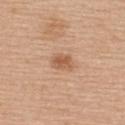Impression:
This lesion was catalogued during total-body skin photography and was not selected for biopsy.
Background:
The total-body-photography lesion software estimated an average lesion color of about L≈58 a*≈21 b*≈34 (CIELAB), roughly 10 lightness units darker than nearby skin, and a lesion-to-skin contrast of about 7 (normalized; higher = more distinct). It also reported an automated nevus-likeness rating near 80 out of 100 and a detector confidence of about 100 out of 100 that the crop contains a lesion. The lesion is on the upper back. The tile uses white-light illumination. A region of skin cropped from a whole-body photographic capture, roughly 15 mm wide. The patient is a female aged 63 to 67. Longest diameter approximately 2.5 mm.